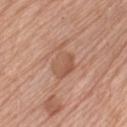Part of a total-body skin-imaging series; this lesion was reviewed on a skin check and was not flagged for biopsy. A close-up tile cropped from a whole-body skin photograph, about 15 mm across. The tile uses white-light illumination. From the left thigh. The subject is a female aged 73–77. Longest diameter approximately 5 mm.